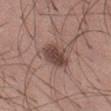Impression: This lesion was catalogued during total-body skin photography and was not selected for biopsy. Clinical summary: The tile uses white-light illumination. A lesion tile, about 15 mm wide, cut from a 3D total-body photograph. About 4 mm across. The lesion-visualizer software estimated an area of roughly 9 mm², an eccentricity of roughly 0.7, and a symmetry-axis asymmetry near 0.2. The software also gave a lesion–skin lightness drop of about 12. And it measured a classifier nevus-likeness of about 75/100. A male subject aged 18 to 22. From the left thigh.